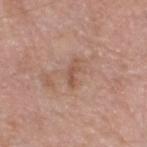* anatomic site · the head or neck
* lesion diameter · ≈3 mm
* lighting · white-light illumination
* patient · male, aged around 65
* imaging modality · total-body-photography crop, ~15 mm field of view